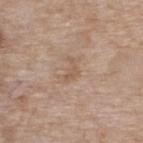{"biopsy_status": "not biopsied; imaged during a skin examination", "site": "upper back", "lesion_size": {"long_diameter_mm_approx": 2.5}, "image": {"source": "total-body photography crop", "field_of_view_mm": 15}, "automated_metrics": {"cielab_L": 56, "cielab_a": 17, "cielab_b": 29, "vs_skin_darker_L": 7.0, "vs_skin_contrast_norm": 5.0, "nevus_likeness_0_100": 0}, "lighting": "white-light", "patient": {"sex": "female", "age_approx": 75}}